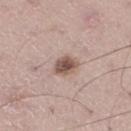Impression:
Part of a total-body skin-imaging series; this lesion was reviewed on a skin check and was not flagged for biopsy.
Clinical summary:
About 3 mm across. The lesion is on the right thigh. A lesion tile, about 15 mm wide, cut from a 3D total-body photograph. A male subject aged approximately 50. Captured under white-light illumination.